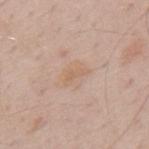biopsy_status: not biopsied; imaged during a skin examination
lighting: white-light
automated_metrics:
  cielab_L: 62
  cielab_a: 17
  cielab_b: 30
  vs_skin_darker_L: 6.0
  vs_skin_contrast_norm: 5.0
  nevus_likeness_0_100: 0
patient:
  sex: male
  age_approx: 70
image:
  source: total-body photography crop
  field_of_view_mm: 15
site: back